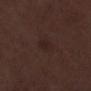Clinical impression: This lesion was catalogued during total-body skin photography and was not selected for biopsy.